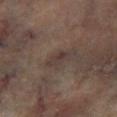Imaged during a routine full-body skin examination; the lesion was not biopsied and no histopathology is available. The lesion's longest dimension is about 3.5 mm. The lesion is on the left lower leg. This is a cross-polarized tile. The patient is a male aged 63–67. A lesion tile, about 15 mm wide, cut from a 3D total-body photograph.